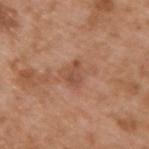{"patient": {"sex": "male", "age_approx": 65}, "lesion_size": {"long_diameter_mm_approx": 3.0}, "site": "upper back", "automated_metrics": {"area_mm2_approx": 3.5, "eccentricity": 0.75, "shape_asymmetry": 0.35, "color_variation_0_10": 2.0, "peripheral_color_asymmetry": 0.5}, "lighting": "white-light", "image": {"source": "total-body photography crop", "field_of_view_mm": 15}}